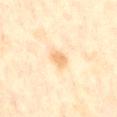Notes:
– notes — imaged on a skin check; not biopsied
– body site — the chest
– acquisition — total-body-photography crop, ~15 mm field of view
– patient — male, roughly 60 years of age